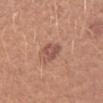  biopsy_status: not biopsied; imaged during a skin examination
  patient:
    sex: female
    age_approx: 25
  site: left forearm
  image:
    source: total-body photography crop
    field_of_view_mm: 15